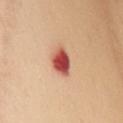Captured during whole-body skin photography for melanoma surveillance; the lesion was not biopsied. The subject is a female aged 58 to 62. A region of skin cropped from a whole-body photographic capture, roughly 15 mm wide. The lesion is on the back.